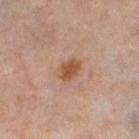Case summary:
- biopsy status — total-body-photography surveillance lesion; no biopsy
- tile lighting — cross-polarized
- location — the leg
- imaging modality — total-body-photography crop, ~15 mm field of view
- subject — female, aged approximately 50